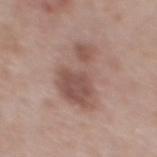Imaged during a routine full-body skin examination; the lesion was not biopsied and no histopathology is available. The tile uses white-light illumination. On the mid back. A close-up tile cropped from a whole-body skin photograph, about 15 mm across. Approximately 7 mm at its widest. The patient is a male aged approximately 55.